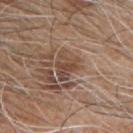Q: Was a biopsy performed?
A: no biopsy performed (imaged during a skin exam)
Q: Lesion size?
A: about 3.5 mm
Q: What lighting was used for the tile?
A: white-light
Q: What kind of image is this?
A: total-body-photography crop, ~15 mm field of view
Q: Lesion location?
A: the front of the torso
Q: What are the patient's age and sex?
A: male, aged approximately 45